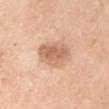Q: Is there a histopathology result?
A: catalogued during a skin exam; not biopsied
Q: Patient demographics?
A: female, roughly 55 years of age
Q: What did automated image analysis measure?
A: a footprint of about 12 mm², a shape eccentricity near 0.6, and a shape-asymmetry score of about 0.2 (0 = symmetric); a border-irregularity index near 2/10, a within-lesion color-variation index near 4/10, and peripheral color asymmetry of about 1.5
Q: Lesion location?
A: the arm
Q: How was this image acquired?
A: ~15 mm crop, total-body skin-cancer survey
Q: What lighting was used for the tile?
A: white-light illumination
Q: What is the lesion's diameter?
A: ≈4 mm